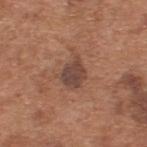follow-up: imaged on a skin check; not biopsied
body site: the upper back
imaging modality: ~15 mm crop, total-body skin-cancer survey
diameter: ~3.5 mm (longest diameter)
automated metrics: an area of roughly 7.5 mm² and an outline eccentricity of about 0.65 (0 = round, 1 = elongated)
patient: male, aged 63 to 67
lighting: white-light illumination